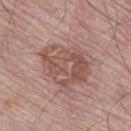notes: catalogued during a skin exam; not biopsied | TBP lesion metrics: a lesion color around L≈52 a*≈20 b*≈24 in CIELAB, roughly 9 lightness units darker than nearby skin, and a lesion-to-skin contrast of about 6.5 (normalized; higher = more distinct); an automated nevus-likeness rating near 0 out of 100 and a detector confidence of about 100 out of 100 that the crop contains a lesion | anatomic site: the left leg | subject: male, aged around 80 | lighting: white-light illumination | imaging modality: total-body-photography crop, ~15 mm field of view | diameter: ≈6 mm.